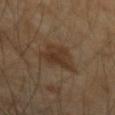The lesion was tiled from a total-body skin photograph and was not biopsied. The patient is a male aged 43–47. A 15 mm close-up extracted from a 3D total-body photography capture. The lesion is located on the left forearm. The tile uses cross-polarized illumination.